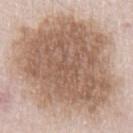Notes:
- biopsy status — no biopsy performed (imaged during a skin exam)
- image — total-body-photography crop, ~15 mm field of view
- size — ~13.5 mm (longest diameter)
- subject — male, aged approximately 65
- location — the arm